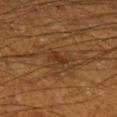Notes:
- workup — imaged on a skin check; not biopsied
- site — the right lower leg
- acquisition — ~15 mm crop, total-body skin-cancer survey
- lesion diameter — about 2.5 mm
- patient — male, in their 60s
- automated lesion analysis — a lesion area of about 4 mm² and a shape eccentricity near 0.75; a color-variation rating of about 2.5/10 and radial color variation of about 1